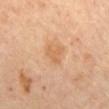notes = imaged on a skin check; not biopsied | illumination = cross-polarized | patient = female, aged around 65 | imaging modality = ~15 mm crop, total-body skin-cancer survey | body site = the mid back | lesion size = about 3 mm | image-analysis metrics = a border-irregularity rating of about 4.5/10, a color-variation rating of about 1.5/10, and peripheral color asymmetry of about 0.5; an automated nevus-likeness rating near 0 out of 100 and a detector confidence of about 100 out of 100 that the crop contains a lesion.